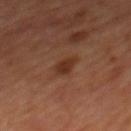Assessment:
Imaged during a routine full-body skin examination; the lesion was not biopsied and no histopathology is available.
Context:
Cropped from a total-body skin-imaging series; the visible field is about 15 mm. Captured under cross-polarized illumination. On the mid back. Measured at roughly 3 mm in maximum diameter. A male subject, aged around 65.